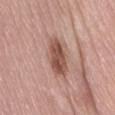Part of a total-body skin-imaging series; this lesion was reviewed on a skin check and was not flagged for biopsy. A male patient, aged 68–72. Captured under white-light illumination. Approximately 5.5 mm at its widest. A roughly 15 mm field-of-view crop from a total-body skin photograph. The total-body-photography lesion software estimated a border-irregularity rating of about 2.5/10 and a peripheral color-asymmetry measure near 1.5. And it measured an automated nevus-likeness rating near 95 out of 100 and a detector confidence of about 100 out of 100 that the crop contains a lesion. On the lower back.